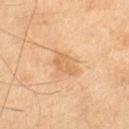Assessment: Imaged during a routine full-body skin examination; the lesion was not biopsied and no histopathology is available. Clinical summary: A male patient about 70 years old. The lesion is located on the left thigh. Automated tile analysis of the lesion measured a footprint of about 5 mm² and two-axis asymmetry of about 0.25. The analysis additionally found about 8 CIELAB-L* units darker than the surrounding skin. The analysis additionally found a border-irregularity index near 3/10, a color-variation rating of about 2/10, and a peripheral color-asymmetry measure near 0.5. A 15 mm close-up extracted from a 3D total-body photography capture. Longest diameter approximately 4 mm. Captured under cross-polarized illumination.